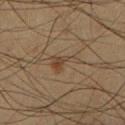The lesion was tiled from a total-body skin photograph and was not biopsied. A 15 mm crop from a total-body photograph taken for skin-cancer surveillance. Longest diameter approximately 3.5 mm. Automated tile analysis of the lesion measured a footprint of about 4.5 mm², a shape eccentricity near 0.85, and two-axis asymmetry of about 0.6. And it measured an average lesion color of about L≈37 a*≈13 b*≈26 (CIELAB), roughly 6 lightness units darker than nearby skin, and a normalized border contrast of about 6. From the right lower leg. A male subject, aged around 35. The tile uses cross-polarized illumination.